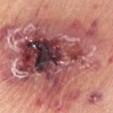tile lighting: white-light
site: the right upper arm
subject: male, roughly 65 years of age
acquisition: ~15 mm crop, total-body skin-cancer survey
image-analysis metrics: about 17 CIELAB-L* units darker than the surrounding skin and a normalized border contrast of about 11.5; border irregularity of about 7.5 on a 0–10 scale, a color-variation rating of about 10/10, and a peripheral color-asymmetry measure near 5; a nevus-likeness score of about 0/100 and a lesion-detection confidence of about 100/100